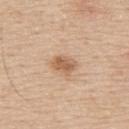Notes:
• workup — total-body-photography surveillance lesion; no biopsy
• automated lesion analysis — roughly 11 lightness units darker than nearby skin; a classifier nevus-likeness of about 75/100 and lesion-presence confidence of about 100/100
• site — the upper back
• subject — male, aged around 55
• imaging modality — ~15 mm tile from a whole-body skin photo
• tile lighting — white-light illumination
• diameter — about 3 mm